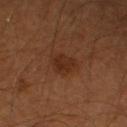Q: Was a biopsy performed?
A: no biopsy performed (imaged during a skin exam)
Q: What are the patient's age and sex?
A: male, aged approximately 70
Q: What is the imaging modality?
A: total-body-photography crop, ~15 mm field of view
Q: What lighting was used for the tile?
A: cross-polarized illumination
Q: Where on the body is the lesion?
A: the right upper arm